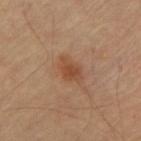Case summary:
- follow-up: total-body-photography surveillance lesion; no biopsy